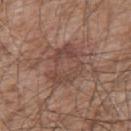notes: total-body-photography surveillance lesion; no biopsy
anatomic site: the upper back
image source: ~15 mm tile from a whole-body skin photo
illumination: white-light illumination
subject: male, aged 53–57